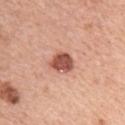| feature | finding |
|---|---|
| workup | catalogued during a skin exam; not biopsied |
| patient | female, aged 58 to 62 |
| lighting | white-light |
| acquisition | ~15 mm crop, total-body skin-cancer survey |
| image-analysis metrics | a lesion area of about 6.5 mm², an eccentricity of roughly 0.55, and a shape-asymmetry score of about 0.25 (0 = symmetric) |
| body site | the left upper arm |
| diameter | ≈3 mm |